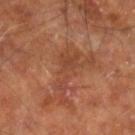Q: Was this lesion biopsied?
A: catalogued during a skin exam; not biopsied
Q: What kind of image is this?
A: total-body-photography crop, ~15 mm field of view
Q: What lighting was used for the tile?
A: cross-polarized
Q: Where on the body is the lesion?
A: the leg
Q: What is the lesion's diameter?
A: ≈5 mm
Q: Automated lesion metrics?
A: an area of roughly 7.5 mm², a shape eccentricity near 0.9, and two-axis asymmetry of about 0.7; a within-lesion color-variation index near 1.5/10 and peripheral color asymmetry of about 0.5; an automated nevus-likeness rating near 0 out of 100 and lesion-presence confidence of about 95/100
Q: What are the patient's age and sex?
A: male, aged 58 to 62